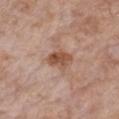Notes:
– biopsy status: no biopsy performed (imaged during a skin exam)